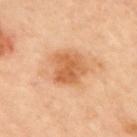Clinical impression:
No biopsy was performed on this lesion — it was imaged during a full skin examination and was not determined to be concerning.
Background:
From the mid back. Automated image analysis of the tile measured a border-irregularity index near 2.5/10 and radial color variation of about 1.5. And it measured a classifier nevus-likeness of about 70/100 and a lesion-detection confidence of about 100/100. A close-up tile cropped from a whole-body skin photograph, about 15 mm across. The recorded lesion diameter is about 4 mm.